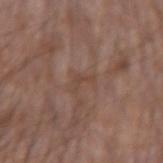workup: total-body-photography surveillance lesion; no biopsy
image: ~15 mm crop, total-body skin-cancer survey
subject: male, aged approximately 55
lesion diameter: ~2.5 mm (longest diameter)
site: the left forearm
illumination: white-light illumination